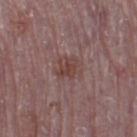{"biopsy_status": "not biopsied; imaged during a skin examination", "lesion_size": {"long_diameter_mm_approx": 4.0}, "site": "left thigh", "patient": {"sex": "male", "age_approx": 75}, "image": {"source": "total-body photography crop", "field_of_view_mm": 15}, "lighting": "white-light"}